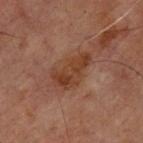workup=total-body-photography surveillance lesion; no biopsy
tile lighting=cross-polarized illumination
patient=male, approximately 70 years of age
acquisition=15 mm crop, total-body photography
location=the chest
diameter=about 5 mm